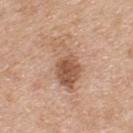{"biopsy_status": "not biopsied; imaged during a skin examination", "lighting": "white-light", "patient": {"sex": "male", "age_approx": 60}, "site": "back", "image": {"source": "total-body photography crop", "field_of_view_mm": 15}, "automated_metrics": {"area_mm2_approx": 13.0, "eccentricity": 0.9, "shape_asymmetry": 0.35}}